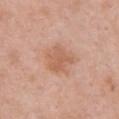Imaged during a routine full-body skin examination; the lesion was not biopsied and no histopathology is available. A female patient aged approximately 40. An algorithmic analysis of the crop reported border irregularity of about 2.5 on a 0–10 scale, a within-lesion color-variation index near 2.5/10, and radial color variation of about 0.5. And it measured a classifier nevus-likeness of about 0/100 and lesion-presence confidence of about 100/100. This is a white-light tile. A 15 mm close-up tile from a total-body photography series done for melanoma screening. The lesion is on the chest. The lesion's longest dimension is about 4 mm.